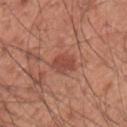Q: Is there a histopathology result?
A: catalogued during a skin exam; not biopsied